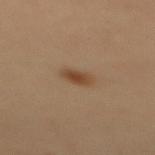Impression:
This lesion was catalogued during total-body skin photography and was not selected for biopsy.
Acquisition and patient details:
A close-up tile cropped from a whole-body skin photograph, about 15 mm across. Captured under cross-polarized illumination. The lesion is located on the upper back. Automated tile analysis of the lesion measured two-axis asymmetry of about 0.2. A female subject, aged around 50.